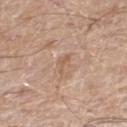{"lesion_size": {"long_diameter_mm_approx": 2.5}, "patient": {"sex": "male", "age_approx": 70}, "site": "right thigh", "image": {"source": "total-body photography crop", "field_of_view_mm": 15}}